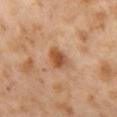Assessment:
Captured during whole-body skin photography for melanoma surveillance; the lesion was not biopsied.
Clinical summary:
From the left thigh. Cropped from a total-body skin-imaging series; the visible field is about 15 mm. This is a cross-polarized tile. A female subject about 55 years old.